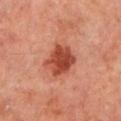follow-up: catalogued during a skin exam; not biopsied | image source: ~15 mm tile from a whole-body skin photo | body site: the left lower leg | diameter: ≈4 mm | tile lighting: cross-polarized illumination | subject: male, about 60 years old.